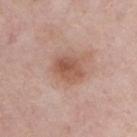Impression: Part of a total-body skin-imaging series; this lesion was reviewed on a skin check and was not flagged for biopsy. Context: A male patient aged 53 to 57. The tile uses white-light illumination. The lesion's longest dimension is about 4 mm. Located on the chest. A roughly 15 mm field-of-view crop from a total-body skin photograph.